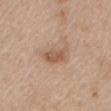workup: catalogued during a skin exam; not biopsied | lighting: white-light illumination | acquisition: 15 mm crop, total-body photography | automated lesion analysis: a footprint of about 7.5 mm², an eccentricity of roughly 0.85, and a symmetry-axis asymmetry near 0.3; a mean CIELAB color near L≈57 a*≈19 b*≈30, roughly 9 lightness units darker than nearby skin, and a lesion-to-skin contrast of about 6.5 (normalized; higher = more distinct); a border-irregularity index near 3.5/10, a color-variation rating of about 4/10, and a peripheral color-asymmetry measure near 1 | body site: the mid back | subject: male, aged 63 to 67.